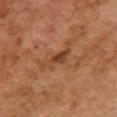Captured during whole-body skin photography for melanoma surveillance; the lesion was not biopsied. About 5.5 mm across. Captured under cross-polarized illumination. The subject is a female aged 58 to 62. A region of skin cropped from a whole-body photographic capture, roughly 15 mm wide. Automated image analysis of the tile measured an average lesion color of about L≈38 a*≈21 b*≈31 (CIELAB), about 8 CIELAB-L* units darker than the surrounding skin, and a lesion-to-skin contrast of about 6.5 (normalized; higher = more distinct). Located on the chest.